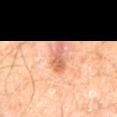Case summary:
• biopsy status · total-body-photography surveillance lesion; no biopsy
• patient · male, about 60 years old
• imaging modality · 15 mm crop, total-body photography
• lesion diameter · ≈5 mm
• lighting · cross-polarized
• TBP lesion metrics · an eccentricity of roughly 0.95 and two-axis asymmetry of about 0.35
• location · the mid back